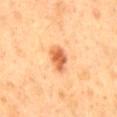Captured during whole-body skin photography for melanoma surveillance; the lesion was not biopsied.
The total-body-photography lesion software estimated a lesion color around L≈62 a*≈29 b*≈42 in CIELAB. The software also gave a classifier nevus-likeness of about 100/100.
Cropped from a whole-body photographic skin survey; the tile spans about 15 mm.
From the back.
The recorded lesion diameter is about 3 mm.
A male patient, aged 43 to 47.
The tile uses cross-polarized illumination.